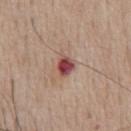workup=no biopsy performed (imaged during a skin exam)
lesion size=~2.5 mm (longest diameter)
automated metrics=a footprint of about 4.5 mm² and a shape eccentricity near 0.55; a border-irregularity index near 2/10 and internal color variation of about 5 on a 0–10 scale
subject=male, roughly 55 years of age
imaging modality=~15 mm tile from a whole-body skin photo
location=the chest
illumination=white-light